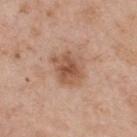subject: male, approximately 55 years of age | image: total-body-photography crop, ~15 mm field of view | anatomic site: the upper back.